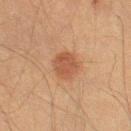Clinical impression: No biopsy was performed on this lesion — it was imaged during a full skin examination and was not determined to be concerning. Image and clinical context: This is a cross-polarized tile. From the right thigh. Cropped from a total-body skin-imaging series; the visible field is about 15 mm. The lesion's longest dimension is about 3.5 mm. A male subject, aged 48 to 52.